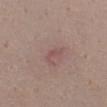<lesion>
<biopsy_status>not biopsied; imaged during a skin examination</biopsy_status>
<lesion_size>
  <long_diameter_mm_approx>2.5</long_diameter_mm_approx>
</lesion_size>
<patient>
  <sex>female</sex>
  <age_approx>35</age_approx>
</patient>
<lighting>white-light</lighting>
<image>
  <source>total-body photography crop</source>
  <field_of_view_mm>15</field_of_view_mm>
</image>
<site>front of the torso</site>
</lesion>